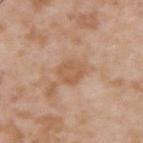follow-up: no biopsy performed (imaged during a skin exam)
tile lighting: white-light illumination
location: the upper back
automated lesion analysis: a mean CIELAB color near L≈57 a*≈20 b*≈33 and a lesion–skin lightness drop of about 8; a within-lesion color-variation index near 2/10; an automated nevus-likeness rating near 0 out of 100 and a detector confidence of about 100 out of 100 that the crop contains a lesion
patient: male, aged 33 to 37
lesion size: ~3 mm (longest diameter)
acquisition: ~15 mm tile from a whole-body skin photo